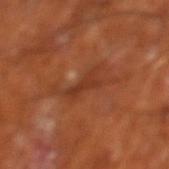Background:
The lesion-visualizer software estimated a lesion area of about 5 mm², an eccentricity of roughly 0.9, and a symmetry-axis asymmetry near 0.5. The analysis additionally found a lesion color around L≈35 a*≈26 b*≈32 in CIELAB and roughly 7 lightness units darker than nearby skin. The analysis additionally found a border-irregularity index near 6.5/10, a color-variation rating of about 0.5/10, and radial color variation of about 0. It also reported lesion-presence confidence of about 75/100. This is a cross-polarized tile. Located on the leg. The lesion's longest dimension is about 4 mm. A male patient aged 63–67. This image is a 15 mm lesion crop taken from a total-body photograph.